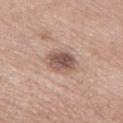From the left thigh. The recorded lesion diameter is about 3.5 mm. Captured under white-light illumination. Automated tile analysis of the lesion measured an average lesion color of about L≈53 a*≈19 b*≈24 (CIELAB), about 13 CIELAB-L* units darker than the surrounding skin, and a lesion-to-skin contrast of about 9 (normalized; higher = more distinct). The analysis additionally found a classifier nevus-likeness of about 45/100 and a detector confidence of about 100 out of 100 that the crop contains a lesion. The subject is a female aged 53 to 57. A 15 mm close-up tile from a total-body photography series done for melanoma screening.